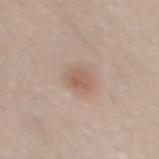notes=catalogued during a skin exam; not biopsied | automated metrics=an average lesion color of about L≈58 a*≈17 b*≈28 (CIELAB), roughly 8 lightness units darker than nearby skin, and a normalized lesion–skin contrast near 6 | body site=the mid back | image source=15 mm crop, total-body photography | patient=male, approximately 35 years of age | diameter=about 2.5 mm | illumination=white-light illumination.